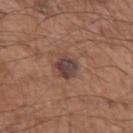The lesion was photographed on a routine skin check and not biopsied; there is no pathology result. A 15 mm close-up tile from a total-body photography series done for melanoma screening. A male subject aged 53 to 57. The lesion is located on the left upper arm. Captured under white-light illumination.